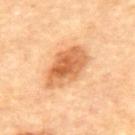Q: Was a biopsy performed?
A: imaged on a skin check; not biopsied
Q: How large is the lesion?
A: about 6 mm
Q: What is the anatomic site?
A: the upper back
Q: Patient demographics?
A: male, about 85 years old or older
Q: What kind of image is this?
A: total-body-photography crop, ~15 mm field of view